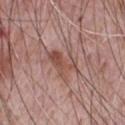Q: Was this lesion biopsied?
A: imaged on a skin check; not biopsied
Q: What kind of image is this?
A: 15 mm crop, total-body photography
Q: What lighting was used for the tile?
A: white-light
Q: How large is the lesion?
A: ~4 mm (longest diameter)
Q: Patient demographics?
A: male, aged approximately 70
Q: Lesion location?
A: the chest